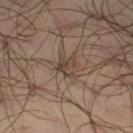Recorded during total-body skin imaging; not selected for excision or biopsy. The recorded lesion diameter is about 3 mm. A male patient, about 65 years old. The lesion is located on the left thigh. An algorithmic analysis of the crop reported a shape eccentricity near 0.6 and a shape-asymmetry score of about 0.3 (0 = symmetric). And it measured a mean CIELAB color near L≈39 a*≈13 b*≈23, about 8 CIELAB-L* units darker than the surrounding skin, and a normalized border contrast of about 7. The software also gave an automated nevus-likeness rating near 5 out of 100 and a lesion-detection confidence of about 80/100. A 15 mm close-up extracted from a 3D total-body photography capture.